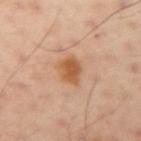notes: catalogued during a skin exam; not biopsied
acquisition: 15 mm crop, total-body photography
anatomic site: the left arm
subject: male, approximately 50 years of age